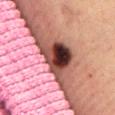The lesion was tiled from a total-body skin photograph and was not biopsied.
From the front of the torso.
Imaged with cross-polarized lighting.
A female patient aged 38–42.
This image is a 15 mm lesion crop taken from a total-body photograph.